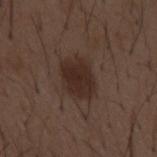workup = no biopsy performed (imaged during a skin exam) | lesion diameter = ~4.5 mm (longest diameter) | patient = male, about 50 years old | anatomic site = the mid back | tile lighting = white-light illumination | acquisition = 15 mm crop, total-body photography.